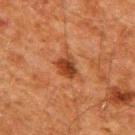Findings:
- follow-up — total-body-photography surveillance lesion; no biopsy
- site — the upper back
- acquisition — ~15 mm tile from a whole-body skin photo
- subject — male, approximately 60 years of age
- automated lesion analysis — a footprint of about 5 mm², an eccentricity of roughly 0.65, and two-axis asymmetry of about 0.25; an average lesion color of about L≈32 a*≈23 b*≈31 (CIELAB) and a normalized border contrast of about 9.5
- illumination — cross-polarized illumination